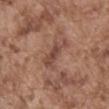Clinical impression:
Captured during whole-body skin photography for melanoma surveillance; the lesion was not biopsied.